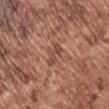- workup: no biopsy performed (imaged during a skin exam)
- subject: male, roughly 75 years of age
- site: the left upper arm
- imaging modality: 15 mm crop, total-body photography